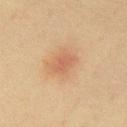Q: Was this lesion biopsied?
A: imaged on a skin check; not biopsied
Q: Illumination type?
A: cross-polarized
Q: What kind of image is this?
A: total-body-photography crop, ~15 mm field of view
Q: What are the patient's age and sex?
A: female, aged around 55
Q: How large is the lesion?
A: ~3 mm (longest diameter)
Q: Automated lesion metrics?
A: a symmetry-axis asymmetry near 0.2; a lesion color around L≈53 a*≈21 b*≈31 in CIELAB, roughly 7 lightness units darker than nearby skin, and a lesion-to-skin contrast of about 5.5 (normalized; higher = more distinct); a nevus-likeness score of about 10/100 and a detector confidence of about 100 out of 100 that the crop contains a lesion
Q: Where on the body is the lesion?
A: the chest